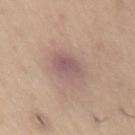The lesion was photographed on a routine skin check and not biopsied; there is no pathology result.
The recorded lesion diameter is about 4.5 mm.
A female subject, aged approximately 40.
Captured under white-light illumination.
On the front of the torso.
A close-up tile cropped from a whole-body skin photograph, about 15 mm across.